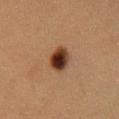Captured during whole-body skin photography for melanoma surveillance; the lesion was not biopsied. The tile uses cross-polarized illumination. The patient is a female about 40 years old. A 15 mm crop from a total-body photograph taken for skin-cancer surveillance. Automated tile analysis of the lesion measured an area of roughly 6.5 mm², an outline eccentricity of about 0.75 (0 = round, 1 = elongated), and a symmetry-axis asymmetry near 0.15. And it measured an average lesion color of about L≈29 a*≈18 b*≈26 (CIELAB), roughly 16 lightness units darker than nearby skin, and a normalized border contrast of about 14.5. Located on the chest. The recorded lesion diameter is about 3.5 mm.